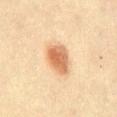Clinical impression:
Part of a total-body skin-imaging series; this lesion was reviewed on a skin check and was not flagged for biopsy.
Image and clinical context:
An algorithmic analysis of the crop reported an area of roughly 9 mm², a shape eccentricity near 0.7, and a symmetry-axis asymmetry near 0.25. And it measured an average lesion color of about L≈57 a*≈20 b*≈34 (CIELAB), a lesion–skin lightness drop of about 13, and a lesion-to-skin contrast of about 9 (normalized; higher = more distinct). It also reported a border-irregularity index near 2/10, a within-lesion color-variation index near 4.5/10, and a peripheral color-asymmetry measure near 1.5. It also reported a nevus-likeness score of about 100/100. A region of skin cropped from a whole-body photographic capture, roughly 15 mm wide. The tile uses cross-polarized illumination. The lesion is located on the abdomen. The recorded lesion diameter is about 4 mm. The subject is a male aged approximately 45.